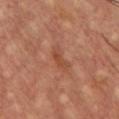Captured during whole-body skin photography for melanoma surveillance; the lesion was not biopsied. Captured under cross-polarized illumination. A roughly 15 mm field-of-view crop from a total-body skin photograph. The lesion is located on the chest. The subject is a male about 55 years old. Automated tile analysis of the lesion measured a normalized border contrast of about 5.5. It also reported a border-irregularity rating of about 4.5/10. It also reported a classifier nevus-likeness of about 0/100 and a detector confidence of about 100 out of 100 that the crop contains a lesion.